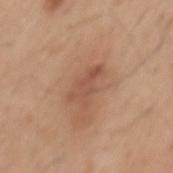body site: the mid back | patient: male, about 45 years old | TBP lesion metrics: a footprint of about 6 mm², an eccentricity of roughly 0.85, and a symmetry-axis asymmetry near 0.4; an automated nevus-likeness rating near 5 out of 100 and lesion-presence confidence of about 100/100 | size: ≈4 mm | acquisition: 15 mm crop, total-body photography.